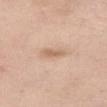– notes — no biopsy performed (imaged during a skin exam)
– subject — female, aged around 55
– automated lesion analysis — a classifier nevus-likeness of about 10/100 and a lesion-detection confidence of about 100/100
– imaging modality — ~15 mm tile from a whole-body skin photo
– site — the right thigh
– size — about 3 mm